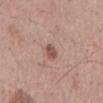Impression:
Captured during whole-body skin photography for melanoma surveillance; the lesion was not biopsied.
Image and clinical context:
A region of skin cropped from a whole-body photographic capture, roughly 15 mm wide. The total-body-photography lesion software estimated an area of roughly 3 mm², an eccentricity of roughly 0.85, and a symmetry-axis asymmetry near 0.25. And it measured an automated nevus-likeness rating near 85 out of 100 and a lesion-detection confidence of about 100/100. From the mid back. The recorded lesion diameter is about 2.5 mm. The subject is a male aged approximately 55.